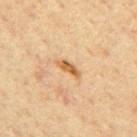{
  "biopsy_status": "not biopsied; imaged during a skin examination",
  "lesion_size": {
    "long_diameter_mm_approx": 3.5
  },
  "site": "mid back",
  "automated_metrics": {
    "eccentricity": 0.9,
    "shape_asymmetry": 0.35,
    "cielab_L": 55,
    "cielab_a": 19,
    "cielab_b": 38,
    "vs_skin_darker_L": 11.0,
    "vs_skin_contrast_norm": 8.5
  },
  "image": {
    "source": "total-body photography crop",
    "field_of_view_mm": 15
  },
  "patient": {
    "sex": "male",
    "age_approx": 65
  },
  "lighting": "cross-polarized"
}